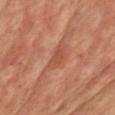The lesion is on the chest. A close-up tile cropped from a whole-body skin photograph, about 15 mm across. The tile uses cross-polarized illumination. The subject is a male aged 68–72.